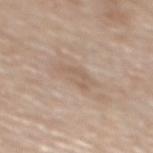{"biopsy_status": "not biopsied; imaged during a skin examination", "lighting": "white-light", "image": {"source": "total-body photography crop", "field_of_view_mm": 15}, "site": "back", "lesion_size": {"long_diameter_mm_approx": 4.0}, "patient": {"sex": "female", "age_approx": 65}}